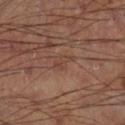Assessment:
Imaged during a routine full-body skin examination; the lesion was not biopsied and no histopathology is available.
Background:
A 15 mm close-up extracted from a 3D total-body photography capture. Located on the right lower leg. Longest diameter approximately 2.5 mm.